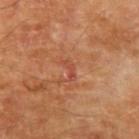The lesion was tiled from a total-body skin photograph and was not biopsied. A male subject aged approximately 70. This is a cross-polarized tile. Located on the left upper arm. The lesion's longest dimension is about 2.5 mm. A close-up tile cropped from a whole-body skin photograph, about 15 mm across.